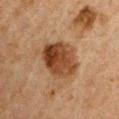biopsy status = imaged on a skin check; not biopsied | image source = total-body-photography crop, ~15 mm field of view | body site = the right upper arm | tile lighting = cross-polarized illumination | TBP lesion metrics = an automated nevus-likeness rating near 85 out of 100 | subject = male, approximately 50 years of age | diameter = ~5 mm (longest diameter).